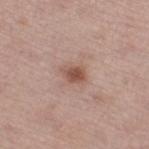The lesion was tiled from a total-body skin photograph and was not biopsied.
Imaged with white-light lighting.
From the right thigh.
The patient is a male about 55 years old.
A close-up tile cropped from a whole-body skin photograph, about 15 mm across.
Measured at roughly 2.5 mm in maximum diameter.
An algorithmic analysis of the crop reported roughly 11 lightness units darker than nearby skin and a normalized border contrast of about 8.